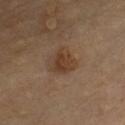Clinical impression:
The lesion was tiled from a total-body skin photograph and was not biopsied.
Acquisition and patient details:
Measured at roughly 3 mm in maximum diameter. The lesion is located on the chest. Automated image analysis of the tile measured an eccentricity of roughly 0.25 and two-axis asymmetry of about 0.25. The software also gave an average lesion color of about L≈39 a*≈17 b*≈29 (CIELAB), a lesion–skin lightness drop of about 8, and a normalized lesion–skin contrast near 7.5. The software also gave an automated nevus-likeness rating near 75 out of 100 and lesion-presence confidence of about 100/100. A male patient about 55 years old. A lesion tile, about 15 mm wide, cut from a 3D total-body photograph. The tile uses cross-polarized illumination.